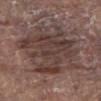<lesion>
<lesion_size>
  <long_diameter_mm_approx>8.5</long_diameter_mm_approx>
</lesion_size>
<site>abdomen</site>
<patient>
  <sex>male</sex>
  <age_approx>80</age_approx>
</patient>
<image>
  <source>total-body photography crop</source>
  <field_of_view_mm>15</field_of_view_mm>
</image>
<lighting>white-light</lighting>
</lesion>